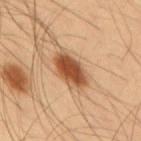Assessment: Captured during whole-body skin photography for melanoma surveillance; the lesion was not biopsied. Context: A 15 mm close-up extracted from a 3D total-body photography capture. Imaged with cross-polarized lighting. The lesion is on the mid back. A male subject, aged around 55. About 5 mm across.